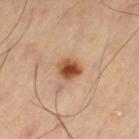Q: Is there a histopathology result?
A: imaged on a skin check; not biopsied
Q: What did automated image analysis measure?
A: a mean CIELAB color near L≈53 a*≈24 b*≈37 and about 15 CIELAB-L* units darker than the surrounding skin; a border-irregularity rating of about 1.5/10, internal color variation of about 6 on a 0–10 scale, and a peripheral color-asymmetry measure near 1.5
Q: Lesion location?
A: the left thigh
Q: What kind of image is this?
A: total-body-photography crop, ~15 mm field of view
Q: Illumination type?
A: cross-polarized illumination